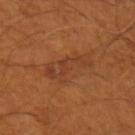Impression: Recorded during total-body skin imaging; not selected for excision or biopsy. Background: The subject is a male aged approximately 55. Automated tile analysis of the lesion measured an automated nevus-likeness rating near 0 out of 100 and lesion-presence confidence of about 100/100. Imaged with cross-polarized lighting. The lesion is on the left upper arm. This image is a 15 mm lesion crop taken from a total-body photograph.